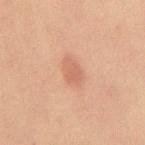follow-up: no biopsy performed (imaged during a skin exam)
automated lesion analysis: an average lesion color of about L≈51 a*≈21 b*≈27 (CIELAB) and a lesion-to-skin contrast of about 5 (normalized; higher = more distinct); a border-irregularity rating of about 3/10, internal color variation of about 2 on a 0–10 scale, and a peripheral color-asymmetry measure near 0.5; an automated nevus-likeness rating near 60 out of 100 and a detector confidence of about 100 out of 100 that the crop contains a lesion
anatomic site: the mid back
diameter: ≈3.5 mm
patient: male, aged 58 to 62
lighting: cross-polarized
image source: ~15 mm tile from a whole-body skin photo